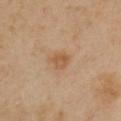Context:
A close-up tile cropped from a whole-body skin photograph, about 15 mm across. The patient is a female aged 38 to 42. Imaged with cross-polarized lighting. On the chest. Automated image analysis of the tile measured a lesion color around L≈56 a*≈20 b*≈35 in CIELAB, about 8 CIELAB-L* units darker than the surrounding skin, and a normalized border contrast of about 6. The recorded lesion diameter is about 2.5 mm.